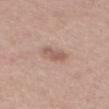<lesion>
  <site>mid back</site>
  <image>
    <source>total-body photography crop</source>
    <field_of_view_mm>15</field_of_view_mm>
  </image>
  <patient>
    <sex>male</sex>
    <age_approx>55</age_approx>
  </patient>
  <lighting>white-light</lighting>
</lesion>